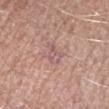Assessment:
Part of a total-body skin-imaging series; this lesion was reviewed on a skin check and was not flagged for biopsy.
Image and clinical context:
A male patient, in their 80s. A close-up tile cropped from a whole-body skin photograph, about 15 mm across. The lesion's longest dimension is about 3 mm. From the arm. This is a white-light tile.